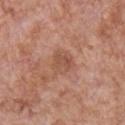The lesion was photographed on a routine skin check and not biopsied; there is no pathology result. The tile uses white-light illumination. The patient is a male about 65 years old. A 15 mm close-up tile from a total-body photography series done for melanoma screening. Located on the chest.